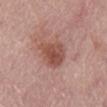The lesion was tiled from a total-body skin photograph and was not biopsied.
A 15 mm crop from a total-body photograph taken for skin-cancer surveillance.
A male patient, aged 73 to 77.
This is a white-light tile.
The lesion-visualizer software estimated an average lesion color of about L≈51 a*≈23 b*≈26 (CIELAB), roughly 10 lightness units darker than nearby skin, and a lesion-to-skin contrast of about 8 (normalized; higher = more distinct). The analysis additionally found lesion-presence confidence of about 100/100.
The lesion is located on the abdomen.